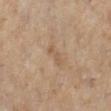This lesion was catalogued during total-body skin photography and was not selected for biopsy.
The total-body-photography lesion software estimated an eccentricity of roughly 0.9 and a symmetry-axis asymmetry near 0.25. It also reported a lesion color around L≈53 a*≈16 b*≈30 in CIELAB, roughly 6 lightness units darker than nearby skin, and a lesion-to-skin contrast of about 4.5 (normalized; higher = more distinct). And it measured border irregularity of about 3.5 on a 0–10 scale, internal color variation of about 0 on a 0–10 scale, and radial color variation of about 0.
The tile uses cross-polarized illumination.
A 15 mm close-up tile from a total-body photography series done for melanoma screening.
The lesion is on the left lower leg.
A female subject in their 50s.